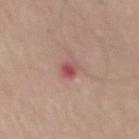Imaged during a routine full-body skin examination; the lesion was not biopsied and no histopathology is available. On the back. A lesion tile, about 15 mm wide, cut from a 3D total-body photograph. A male subject aged 53–57. Captured under white-light illumination. Approximately 3 mm at its widest.